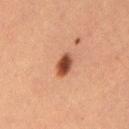The lesion's longest dimension is about 3 mm. The lesion is located on the leg. A close-up tile cropped from a whole-body skin photograph, about 15 mm across. A female patient, in their 40s.